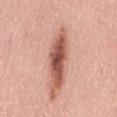Imaged during a routine full-body skin examination; the lesion was not biopsied and no histopathology is available.
Imaged with white-light lighting.
Measured at roughly 3.5 mm in maximum diameter.
This image is a 15 mm lesion crop taken from a total-body photograph.
On the back.
Automated tile analysis of the lesion measured a lesion color around L≈48 a*≈27 b*≈27 in CIELAB, about 20 CIELAB-L* units darker than the surrounding skin, and a lesion-to-skin contrast of about 12.5 (normalized; higher = more distinct). The software also gave border irregularity of about 3.5 on a 0–10 scale, internal color variation of about 1.5 on a 0–10 scale, and peripheral color asymmetry of about 0.5. The software also gave a nevus-likeness score of about 0/100 and a detector confidence of about 15 out of 100 that the crop contains a lesion.
The patient is a male aged 53 to 57.